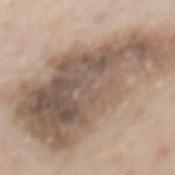The lesion was tiled from a total-body skin photograph and was not biopsied.
Automated image analysis of the tile measured an area of roughly 70 mm², an outline eccentricity of about 0.9 (0 = round, 1 = elongated), and a shape-asymmetry score of about 0.4 (0 = symmetric).
Cropped from a whole-body photographic skin survey; the tile spans about 15 mm.
This is a white-light tile.
Located on the mid back.
A male subject, about 55 years old.
Measured at roughly 14.5 mm in maximum diameter.